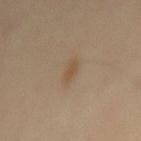{"biopsy_status": "not biopsied; imaged during a skin examination", "automated_metrics": {"area_mm2_approx": 3.5, "eccentricity": 0.9, "shape_asymmetry": 0.1, "cielab_L": 51, "cielab_a": 13, "cielab_b": 31, "vs_skin_darker_L": 6.0, "vs_skin_contrast_norm": 5.5, "lesion_detection_confidence_0_100": 100}, "patient": {"sex": "male", "age_approx": 55}, "lesion_size": {"long_diameter_mm_approx": 3.0}, "lighting": "cross-polarized", "image": {"source": "total-body photography crop", "field_of_view_mm": 15}, "site": "mid back"}